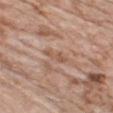Impression:
Imaged during a routine full-body skin examination; the lesion was not biopsied and no histopathology is available.
Clinical summary:
A 15 mm close-up extracted from a 3D total-body photography capture. A male subject, in their mid- to late 70s. The lesion is on the chest.